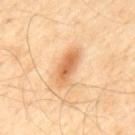The lesion was tiled from a total-body skin photograph and was not biopsied. The subject is a male aged around 55. A 15 mm close-up extracted from a 3D total-body photography capture. On the mid back.